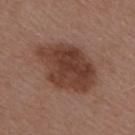Imaged during a routine full-body skin examination; the lesion was not biopsied and no histopathology is available. A lesion tile, about 15 mm wide, cut from a 3D total-body photograph. A female patient, aged around 45. About 7.5 mm across. The lesion is on the right upper arm. This is a white-light tile.